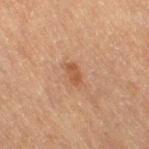Assessment:
Part of a total-body skin-imaging series; this lesion was reviewed on a skin check and was not flagged for biopsy.
Background:
Cropped from a total-body skin-imaging series; the visible field is about 15 mm. Longest diameter approximately 2.5 mm. The patient is a female aged 53–57. An algorithmic analysis of the crop reported an area of roughly 2.5 mm², a shape eccentricity near 0.85, and a symmetry-axis asymmetry near 0.35. From the right thigh. Imaged with cross-polarized lighting.